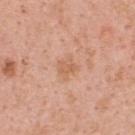Part of a total-body skin-imaging series; this lesion was reviewed on a skin check and was not flagged for biopsy.